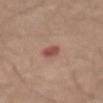{"biopsy_status": "not biopsied; imaged during a skin examination"}